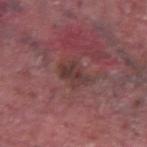| feature | finding |
|---|---|
| workup | total-body-photography surveillance lesion; no biopsy |
| size | ~3.5 mm (longest diameter) |
| image | ~15 mm tile from a whole-body skin photo |
| illumination | white-light illumination |
| subject | male, aged 38 to 42 |
| site | the right upper arm |
| TBP lesion metrics | an area of roughly 5.5 mm² and a shape-asymmetry score of about 0.3 (0 = symmetric); a mean CIELAB color near L≈36 a*≈21 b*≈19, roughly 8 lightness units darker than nearby skin, and a normalized lesion–skin contrast near 7 |